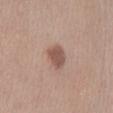Clinical impression: No biopsy was performed on this lesion — it was imaged during a full skin examination and was not determined to be concerning.